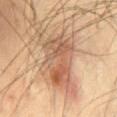notes: imaged on a skin check; not biopsied
imaging modality: ~15 mm tile from a whole-body skin photo
automated lesion analysis: border irregularity of about 5.5 on a 0–10 scale and peripheral color asymmetry of about 2.5; a nevus-likeness score of about 75/100 and a detector confidence of about 100 out of 100 that the crop contains a lesion
site: the abdomen
patient: male, roughly 40 years of age